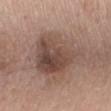Q: Was a biopsy performed?
A: catalogued during a skin exam; not biopsied
Q: Who is the patient?
A: female, aged around 60
Q: Where on the body is the lesion?
A: the mid back
Q: What kind of image is this?
A: total-body-photography crop, ~15 mm field of view